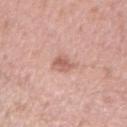{"biopsy_status": "not biopsied; imaged during a skin examination", "lighting": "white-light", "site": "arm", "patient": {"sex": "male", "age_approx": 35}, "image": {"source": "total-body photography crop", "field_of_view_mm": 15}, "lesion_size": {"long_diameter_mm_approx": 2.5}}